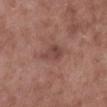An algorithmic analysis of the crop reported a footprint of about 5 mm², an outline eccentricity of about 0.75 (0 = round, 1 = elongated), and a symmetry-axis asymmetry near 0.25. The analysis additionally found a lesion color around L≈44 a*≈21 b*≈24 in CIELAB, a lesion–skin lightness drop of about 8, and a normalized border contrast of about 6.5. A 15 mm crop from a total-body photograph taken for skin-cancer surveillance. From the left lower leg. The tile uses white-light illumination. The subject is a male aged around 55. Approximately 3.5 mm at its widest.